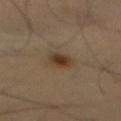workup: no biopsy performed (imaged during a skin exam)
location: the front of the torso
acquisition: 15 mm crop, total-body photography
automated lesion analysis: a footprint of about 4.5 mm², an eccentricity of roughly 0.6, and a symmetry-axis asymmetry near 0.25; a mean CIELAB color near L≈34 a*≈14 b*≈27; a color-variation rating of about 3/10 and radial color variation of about 0.5; a detector confidence of about 100 out of 100 that the crop contains a lesion
patient: male, aged 53–57
illumination: cross-polarized illumination
lesion diameter: ≈2.5 mm